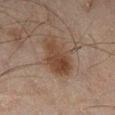Clinical impression:
Recorded during total-body skin imaging; not selected for excision or biopsy.
Clinical summary:
This image is a 15 mm lesion crop taken from a total-body photograph. The lesion is located on the right lower leg. A male patient aged 43 to 47. Approximately 5 mm at its widest. An algorithmic analysis of the crop reported a footprint of about 13 mm², an outline eccentricity of about 0.8 (0 = round, 1 = elongated), and a symmetry-axis asymmetry near 0.3. It also reported an average lesion color of about L≈34 a*≈15 b*≈23 (CIELAB) and a lesion-to-skin contrast of about 8 (normalized; higher = more distinct). The analysis additionally found a color-variation rating of about 4/10 and a peripheral color-asymmetry measure near 1.5. The software also gave a classifier nevus-likeness of about 75/100 and lesion-presence confidence of about 100/100.